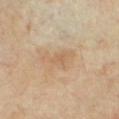notes — catalogued during a skin exam; not biopsied
body site — the chest
tile lighting — cross-polarized
subject — male, aged approximately 65
lesion size — ~4 mm (longest diameter)
acquisition — ~15 mm tile from a whole-body skin photo